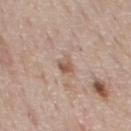The lesion was tiled from a total-body skin photograph and was not biopsied.
The lesion-visualizer software estimated an area of roughly 3 mm² and two-axis asymmetry of about 0.2. The software also gave an automated nevus-likeness rating near 0 out of 100 and lesion-presence confidence of about 100/100.
Captured under white-light illumination.
A male subject, aged approximately 75.
Longest diameter approximately 2.5 mm.
The lesion is on the chest.
A 15 mm crop from a total-body photograph taken for skin-cancer surveillance.